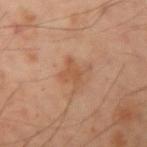Part of a total-body skin-imaging series; this lesion was reviewed on a skin check and was not flagged for biopsy. This is a cross-polarized tile. A male patient, aged 48–52. The recorded lesion diameter is about 3 mm. The lesion is on the left arm. Automated tile analysis of the lesion measured an area of roughly 6 mm² and two-axis asymmetry of about 0.35. And it measured a border-irregularity index near 5.5/10, a within-lesion color-variation index near 1/10, and a peripheral color-asymmetry measure near 0.5. And it measured an automated nevus-likeness rating near 5 out of 100 and a detector confidence of about 100 out of 100 that the crop contains a lesion. A 15 mm close-up extracted from a 3D total-body photography capture.